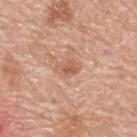Clinical summary:
A male subject, aged approximately 80. Captured under white-light illumination. The lesion is located on the upper back. Cropped from a whole-body photographic skin survey; the tile spans about 15 mm.